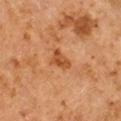Recorded during total-body skin imaging; not selected for excision or biopsy. The total-body-photography lesion software estimated a lesion area of about 4.5 mm² and an outline eccentricity of about 0.75 (0 = round, 1 = elongated). The analysis additionally found a lesion color around L≈49 a*≈27 b*≈40 in CIELAB, about 10 CIELAB-L* units darker than the surrounding skin, and a lesion-to-skin contrast of about 7.5 (normalized; higher = more distinct). And it measured a peripheral color-asymmetry measure near 1. And it measured a detector confidence of about 100 out of 100 that the crop contains a lesion. A male patient roughly 60 years of age. This is a cross-polarized tile. Located on the right arm. This image is a 15 mm lesion crop taken from a total-body photograph. The recorded lesion diameter is about 3 mm.